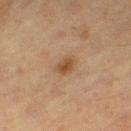No biopsy was performed on this lesion — it was imaged during a full skin examination and was not determined to be concerning. Measured at roughly 2.5 mm in maximum diameter. Cropped from a whole-body photographic skin survey; the tile spans about 15 mm. The tile uses cross-polarized illumination. The lesion is on the leg. A female subject aged around 55.